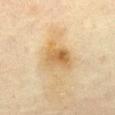Impression: Captured during whole-body skin photography for melanoma surveillance; the lesion was not biopsied. Context: From the front of the torso. Captured under cross-polarized illumination. An algorithmic analysis of the crop reported a mean CIELAB color near L≈54 a*≈13 b*≈36, roughly 9 lightness units darker than nearby skin, and a normalized border contrast of about 7. It also reported an automated nevus-likeness rating near 5 out of 100 and a lesion-detection confidence of about 100/100. The patient is a male aged 73 to 77. A 15 mm close-up extracted from a 3D total-body photography capture.